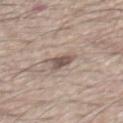Q: Was a biopsy performed?
A: imaged on a skin check; not biopsied
Q: What is the lesion's diameter?
A: ~3 mm (longest diameter)
Q: Where on the body is the lesion?
A: the mid back
Q: What is the imaging modality?
A: ~15 mm crop, total-body skin-cancer survey
Q: What are the patient's age and sex?
A: male, aged around 65